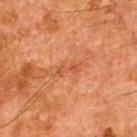Q: How was this image acquired?
A: 15 mm crop, total-body photography
Q: What are the patient's age and sex?
A: male, approximately 65 years of age
Q: How was the tile lit?
A: cross-polarized
Q: What did automated image analysis measure?
A: a lesion area of about 3.5 mm², an outline eccentricity of about 0.9 (0 = round, 1 = elongated), and a shape-asymmetry score of about 0.45 (0 = symmetric); a border-irregularity index near 6/10 and internal color variation of about 0.5 on a 0–10 scale; an automated nevus-likeness rating near 0 out of 100
Q: Where on the body is the lesion?
A: the upper back
Q: Lesion size?
A: ~3.5 mm (longest diameter)